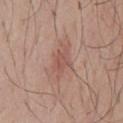<tbp_lesion>
<biopsy_status>not biopsied; imaged during a skin examination</biopsy_status>
<automated_metrics>
  <eccentricity>0.75</eccentricity>
  <shape_asymmetry>0.35</shape_asymmetry>
  <nevus_likeness_0_100>15</nevus_likeness_0_100>
</automated_metrics>
<image>
  <source>total-body photography crop</source>
  <field_of_view_mm>15</field_of_view_mm>
</image>
<site>chest</site>
<patient>
  <sex>male</sex>
  <age_approx>45</age_approx>
</patient>
<lighting>white-light</lighting>
</tbp_lesion>